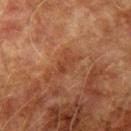Imaged during a routine full-body skin examination; the lesion was not biopsied and no histopathology is available.
From the arm.
The subject is a male approximately 75 years of age.
Cropped from a whole-body photographic skin survey; the tile spans about 15 mm.